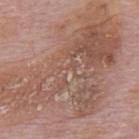Q: Is there a histopathology result?
A: no biopsy performed (imaged during a skin exam)
Q: What is the imaging modality?
A: 15 mm crop, total-body photography
Q: How was the tile lit?
A: white-light
Q: What are the patient's age and sex?
A: male, in their mid- to late 70s
Q: What is the anatomic site?
A: the mid back